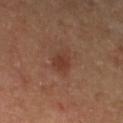Notes:
• workup — total-body-photography surveillance lesion; no biopsy
• anatomic site — the left lower leg
• lighting — cross-polarized illumination
• image — ~15 mm tile from a whole-body skin photo
• patient — male, roughly 65 years of age